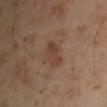Q: Is there a histopathology result?
A: no biopsy performed (imaged during a skin exam)
Q: What is the lesion's diameter?
A: about 3.5 mm
Q: Illumination type?
A: cross-polarized illumination
Q: Automated lesion metrics?
A: an area of roughly 5 mm² and a shape-asymmetry score of about 0.3 (0 = symmetric); border irregularity of about 3.5 on a 0–10 scale, a color-variation rating of about 3/10, and a peripheral color-asymmetry measure near 1; a detector confidence of about 100 out of 100 that the crop contains a lesion
Q: Lesion location?
A: the left upper arm
Q: Who is the patient?
A: male, roughly 45 years of age
Q: How was this image acquired?
A: ~15 mm crop, total-body skin-cancer survey